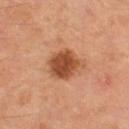Recorded during total-body skin imaging; not selected for excision or biopsy. Located on the left thigh. A male subject aged 53–57. A 15 mm close-up extracted from a 3D total-body photography capture.